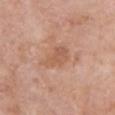Background: Imaged with white-light lighting. About 3.5 mm across. A female subject aged approximately 75. Automated image analysis of the tile measured a footprint of about 6.5 mm², a shape eccentricity near 0.75, and two-axis asymmetry of about 0.35. The analysis additionally found a mean CIELAB color near L≈57 a*≈22 b*≈32 and about 7 CIELAB-L* units darker than the surrounding skin. The software also gave an automated nevus-likeness rating near 0 out of 100 and a detector confidence of about 100 out of 100 that the crop contains a lesion. The lesion is on the chest. A lesion tile, about 15 mm wide, cut from a 3D total-body photograph.